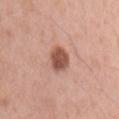biopsy status: imaged on a skin check; not biopsied | image-analysis metrics: an average lesion color of about L≈53 a*≈24 b*≈28 (CIELAB) and a lesion–skin lightness drop of about 15; a border-irregularity rating of about 1.5/10 and a within-lesion color-variation index near 3/10; an automated nevus-likeness rating near 80 out of 100 and lesion-presence confidence of about 100/100 | imaging modality: ~15 mm tile from a whole-body skin photo | subject: male, aged approximately 55 | location: the left upper arm | lesion size: ≈3.5 mm | lighting: white-light illumination.